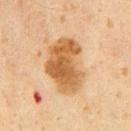biopsy status = no biopsy performed (imaged during a skin exam)
TBP lesion metrics = an area of roughly 22 mm², a shape eccentricity near 0.75, and two-axis asymmetry of about 0.3; a mean CIELAB color near L≈50 a*≈18 b*≈36, roughly 12 lightness units darker than nearby skin, and a normalized lesion–skin contrast near 9.5; a within-lesion color-variation index near 5/10
site = the chest
imaging modality = 15 mm crop, total-body photography
tile lighting = cross-polarized
subject = male, roughly 60 years of age